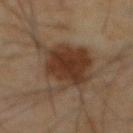notes=imaged on a skin check; not biopsied | image=~15 mm crop, total-body skin-cancer survey | site=the abdomen | tile lighting=cross-polarized | patient=male, in their mid-60s.